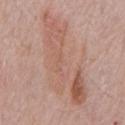notes = total-body-photography surveillance lesion; no biopsy
patient = male, aged 73 to 77
image source = ~15 mm tile from a whole-body skin photo
lesion diameter = ≈12 mm
lighting = white-light
body site = the front of the torso